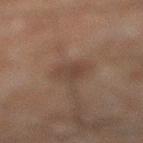Q: Is there a histopathology result?
A: imaged on a skin check; not biopsied
Q: How was this image acquired?
A: ~15 mm crop, total-body skin-cancer survey
Q: Automated lesion metrics?
A: a border-irregularity rating of about 4/10 and radial color variation of about 0.5
Q: Who is the patient?
A: male, about 60 years old
Q: Where on the body is the lesion?
A: the left leg